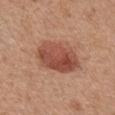| feature | finding |
|---|---|
| biopsy status | no biopsy performed (imaged during a skin exam) |
| image-analysis metrics | a border-irregularity rating of about 1.5/10, internal color variation of about 5.5 on a 0–10 scale, and a peripheral color-asymmetry measure near 2; a classifier nevus-likeness of about 100/100 |
| site | the chest |
| subject | male, roughly 65 years of age |
| diameter | ≈5.5 mm |
| lighting | white-light illumination |
| imaging modality | ~15 mm tile from a whole-body skin photo |